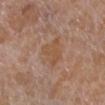{"biopsy_status": "not biopsied; imaged during a skin examination", "site": "right lower leg", "lesion_size": {"long_diameter_mm_approx": 3.5}, "image": {"source": "total-body photography crop", "field_of_view_mm": 15}, "automated_metrics": {"cielab_L": 52, "cielab_a": 19, "cielab_b": 32, "vs_skin_darker_L": 6.0}, "patient": {"sex": "female", "age_approx": 55}, "lighting": "white-light"}